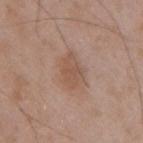Q: Is there a histopathology result?
A: catalogued during a skin exam; not biopsied
Q: What did automated image analysis measure?
A: two-axis asymmetry of about 0.3; a border-irregularity rating of about 3/10, a color-variation rating of about 2/10, and peripheral color asymmetry of about 0.5; a classifier nevus-likeness of about 15/100 and a lesion-detection confidence of about 100/100
Q: How was the tile lit?
A: white-light
Q: Where on the body is the lesion?
A: the back
Q: How large is the lesion?
A: about 4 mm
Q: Patient demographics?
A: male, aged around 50
Q: What is the imaging modality?
A: total-body-photography crop, ~15 mm field of view